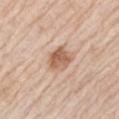Image and clinical context:
About 3.5 mm across. A 15 mm close-up tile from a total-body photography series done for melanoma screening. Captured under white-light illumination. A male patient, aged approximately 80. The total-body-photography lesion software estimated a border-irregularity rating of about 2/10, a within-lesion color-variation index near 4/10, and a peripheral color-asymmetry measure near 1.5. And it measured an automated nevus-likeness rating near 75 out of 100 and a detector confidence of about 100 out of 100 that the crop contains a lesion. From the left upper arm.